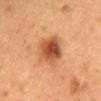| field | value |
|---|---|
| biopsy status | total-body-photography surveillance lesion; no biopsy |
| lighting | cross-polarized |
| patient | female, aged approximately 50 |
| diameter | ~5 mm (longest diameter) |
| TBP lesion metrics | a nevus-likeness score of about 95/100 and lesion-presence confidence of about 100/100 |
| site | the mid back |
| imaging modality | ~15 mm crop, total-body skin-cancer survey |